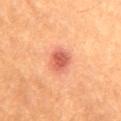biopsy_status: not biopsied; imaged during a skin examination